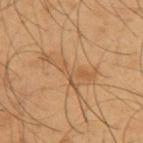workup = imaged on a skin check; not biopsied
subject = male, aged approximately 55
image = ~15 mm crop, total-body skin-cancer survey
anatomic site = the upper back